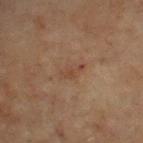The lesion was photographed on a routine skin check and not biopsied; there is no pathology result. Longest diameter approximately 3 mm. A male subject about 70 years old. Located on the front of the torso. A lesion tile, about 15 mm wide, cut from a 3D total-body photograph.